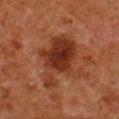<tbp_lesion>
  <biopsy_status>not biopsied; imaged during a skin examination</biopsy_status>
  <patient>
    <sex>male</sex>
    <age_approx>80</age_approx>
  </patient>
  <image>
    <source>total-body photography crop</source>
    <field_of_view_mm>15</field_of_view_mm>
  </image>
  <lesion_size>
    <long_diameter_mm_approx>6.5</long_diameter_mm_approx>
  </lesion_size>
  <automated_metrics>
    <vs_skin_darker_L>9.0</vs_skin_darker_L>
    <vs_skin_contrast_norm>9.0</vs_skin_contrast_norm>
    <border_irregularity_0_10>6.0</border_irregularity_0_10>
    <color_variation_0_10>4.5</color_variation_0_10>
    <peripheral_color_asymmetry>1.5</peripheral_color_asymmetry>
  </automated_metrics>
  <site>right lower leg</site>
  <lighting>cross-polarized</lighting>
</tbp_lesion>